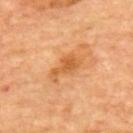Impression:
Captured during whole-body skin photography for melanoma surveillance; the lesion was not biopsied.
Context:
A subject aged approximately 65. Cropped from a whole-body photographic skin survey; the tile spans about 15 mm. Measured at roughly 4 mm in maximum diameter. Located on the upper back.